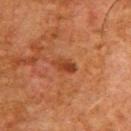Assessment: Imaged during a routine full-body skin examination; the lesion was not biopsied and no histopathology is available. Acquisition and patient details: Measured at roughly 3 mm in maximum diameter. A male subject aged around 65. The tile uses cross-polarized illumination. Located on the back. A 15 mm crop from a total-body photograph taken for skin-cancer surveillance.